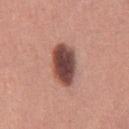Impression:
Captured during whole-body skin photography for melanoma surveillance; the lesion was not biopsied.
Clinical summary:
This image is a 15 mm lesion crop taken from a total-body photograph. A female patient aged 28 to 32. The lesion is located on the right thigh. Imaged with white-light lighting.